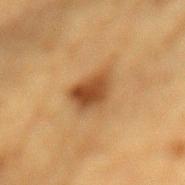{
  "biopsy_status": "not biopsied; imaged during a skin examination",
  "image": {
    "source": "total-body photography crop",
    "field_of_view_mm": 15
  },
  "site": "mid back",
  "lesion_size": {
    "long_diameter_mm_approx": 4.0
  },
  "lighting": "cross-polarized",
  "patient": {
    "sex": "male",
    "age_approx": "85+"
  }
}